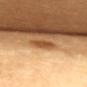Impression: The lesion was photographed on a routine skin check and not biopsied; there is no pathology result. Image and clinical context: On the chest. A female patient, aged 63–67. Cropped from a total-body skin-imaging series; the visible field is about 15 mm. Imaged with cross-polarized lighting. The total-body-photography lesion software estimated a classifier nevus-likeness of about 80/100. Longest diameter approximately 2.5 mm.